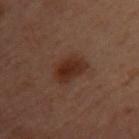notes: catalogued during a skin exam; not biopsied